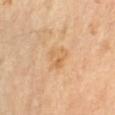follow-up=no biopsy performed (imaged during a skin exam) | body site=the left forearm | acquisition=~15 mm crop, total-body skin-cancer survey | illumination=cross-polarized illumination | patient=female, in their mid- to late 60s | automated lesion analysis=a lesion area of about 7 mm² and an eccentricity of roughly 0.5; a mean CIELAB color near L≈67 a*≈19 b*≈41, a lesion–skin lightness drop of about 7, and a normalized lesion–skin contrast near 5; a border-irregularity rating of about 3/10 and a color-variation rating of about 4.5/10; an automated nevus-likeness rating near 0 out of 100 and a lesion-detection confidence of about 100/100.